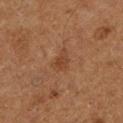Recorded during total-body skin imaging; not selected for excision or biopsy.